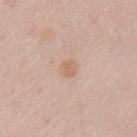<record>
  <image>
    <source>total-body photography crop</source>
    <field_of_view_mm>15</field_of_view_mm>
  </image>
  <automated_metrics>
    <area_mm2_approx>4.0</area_mm2_approx>
    <eccentricity>0.65</eccentricity>
    <shape_asymmetry>0.15</shape_asymmetry>
    <border_irregularity_0_10>1.5</border_irregularity_0_10>
    <color_variation_0_10>2.0</color_variation_0_10>
    <peripheral_color_asymmetry>0.5</peripheral_color_asymmetry>
    <nevus_likeness_0_100>5</nevus_likeness_0_100>
    <lesion_detection_confidence_0_100>100</lesion_detection_confidence_0_100>
  </automated_metrics>
  <site>left upper arm</site>
  <lighting>white-light</lighting>
  <lesion_size>
    <long_diameter_mm_approx>2.5</long_diameter_mm_approx>
  </lesion_size>
  <patient>
    <sex>female</sex>
    <age_approx>50</age_approx>
  </patient>
</record>